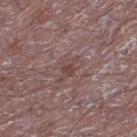{
  "biopsy_status": "not biopsied; imaged during a skin examination",
  "automated_metrics": {
    "vs_skin_darker_L": 7.0,
    "vs_skin_contrast_norm": 6.0,
    "nevus_likeness_0_100": 0
  },
  "lighting": "white-light",
  "image": {
    "source": "total-body photography crop",
    "field_of_view_mm": 15
  },
  "lesion_size": {
    "long_diameter_mm_approx": 3.5
  },
  "patient": {
    "sex": "male",
    "age_approx": 50
  },
  "site": "leg"
}